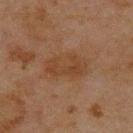Part of a total-body skin-imaging series; this lesion was reviewed on a skin check and was not flagged for biopsy. A region of skin cropped from a whole-body photographic capture, roughly 15 mm wide. From the upper back. A male subject about 45 years old. The tile uses cross-polarized illumination. The recorded lesion diameter is about 5 mm. The lesion-visualizer software estimated a footprint of about 12 mm², an eccentricity of roughly 0.8, and a shape-asymmetry score of about 0.2 (0 = symmetric). The software also gave a classifier nevus-likeness of about 0/100 and a detector confidence of about 100 out of 100 that the crop contains a lesion.